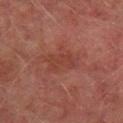The lesion was tiled from a total-body skin photograph and was not biopsied.
From the right lower leg.
A 15 mm close-up tile from a total-body photography series done for melanoma screening.
Longest diameter approximately 4.5 mm.
Automated image analysis of the tile measured border irregularity of about 3.5 on a 0–10 scale and internal color variation of about 2 on a 0–10 scale.
Imaged with cross-polarized lighting.
A male subject, aged approximately 75.